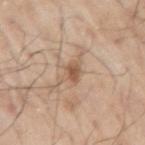Imaged during a routine full-body skin examination; the lesion was not biopsied and no histopathology is available.
The lesion's longest dimension is about 4 mm.
A male patient approximately 70 years of age.
Cropped from a whole-body photographic skin survey; the tile spans about 15 mm.
On the right upper arm.
Captured under white-light illumination.
Automated tile analysis of the lesion measured an eccentricity of roughly 0.85. The software also gave an automated nevus-likeness rating near 30 out of 100 and a detector confidence of about 100 out of 100 that the crop contains a lesion.